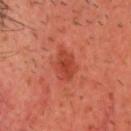  biopsy_status: not biopsied; imaged during a skin examination
  image:
    source: total-body photography crop
    field_of_view_mm: 15
  patient:
    sex: male
    age_approx: 50
  site: head or neck